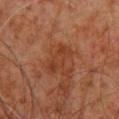Clinical impression:
No biopsy was performed on this lesion — it was imaged during a full skin examination and was not determined to be concerning.
Background:
The lesion is located on the upper back. A lesion tile, about 15 mm wide, cut from a 3D total-body photograph. The subject is a male approximately 60 years of age. Measured at roughly 4 mm in maximum diameter. This is a cross-polarized tile. The lesion-visualizer software estimated roughly 6 lightness units darker than nearby skin and a lesion-to-skin contrast of about 5.5 (normalized; higher = more distinct). And it measured internal color variation of about 3 on a 0–10 scale and radial color variation of about 1. It also reported a nevus-likeness score of about 0/100 and a detector confidence of about 100 out of 100 that the crop contains a lesion.